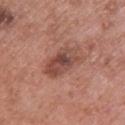Assessment:
The lesion was photographed on a routine skin check and not biopsied; there is no pathology result.
Image and clinical context:
From the upper back. The recorded lesion diameter is about 5 mm. Imaged with white-light lighting. This image is a 15 mm lesion crop taken from a total-body photograph. The subject is a female aged 58 to 62.